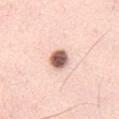Recorded during total-body skin imaging; not selected for excision or biopsy. On the chest. Captured under white-light illumination. A male subject, approximately 35 years of age. A region of skin cropped from a whole-body photographic capture, roughly 15 mm wide. Automated tile analysis of the lesion measured an average lesion color of about L≈62 a*≈21 b*≈26 (CIELAB), about 23 CIELAB-L* units darker than the surrounding skin, and a normalized lesion–skin contrast near 13. It also reported border irregularity of about 1.5 on a 0–10 scale, a color-variation rating of about 5/10, and radial color variation of about 1.5. It also reported a classifier nevus-likeness of about 100/100. Measured at roughly 3 mm in maximum diameter.